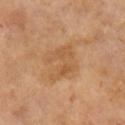Imaged during a routine full-body skin examination; the lesion was not biopsied and no histopathology is available. The total-body-photography lesion software estimated a border-irregularity rating of about 7.5/10, internal color variation of about 2.5 on a 0–10 scale, and radial color variation of about 1. The lesion is on the arm. The patient is a male about 65 years old. The lesion's longest dimension is about 4 mm. A 15 mm crop from a total-body photograph taken for skin-cancer surveillance. The tile uses cross-polarized illumination.